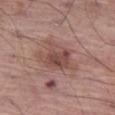Q: Was this lesion biopsied?
A: imaged on a skin check; not biopsied
Q: What is the imaging modality?
A: ~15 mm tile from a whole-body skin photo
Q: Who is the patient?
A: male, aged around 65
Q: What is the anatomic site?
A: the left thigh